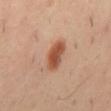Captured during whole-body skin photography for melanoma surveillance; the lesion was not biopsied.
The lesion's longest dimension is about 4 mm.
On the mid back.
A lesion tile, about 15 mm wide, cut from a 3D total-body photograph.
A male patient aged 38 to 42.
Imaged with cross-polarized lighting.